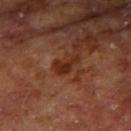notes: total-body-photography surveillance lesion; no biopsy | patient: male, approximately 65 years of age | body site: the right upper arm | lesion diameter: about 3 mm | image source: total-body-photography crop, ~15 mm field of view | tile lighting: cross-polarized illumination.